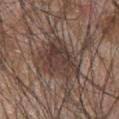{"biopsy_status": "not biopsied; imaged during a skin examination", "image": {"source": "total-body photography crop", "field_of_view_mm": 15}, "site": "upper back", "patient": {"sex": "male", "age_approx": 45}}